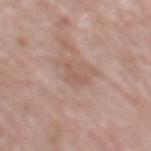Clinical impression:
This lesion was catalogued during total-body skin photography and was not selected for biopsy.
Acquisition and patient details:
The lesion is on the back. A female subject, roughly 60 years of age. A 15 mm crop from a total-body photograph taken for skin-cancer surveillance.